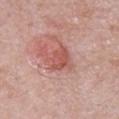The lesion was tiled from a total-body skin photograph and was not biopsied.
The lesion is on the chest.
A male patient in their mid- to late 50s.
A 15 mm crop from a total-body photograph taken for skin-cancer surveillance.